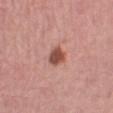Q: Is there a histopathology result?
A: imaged on a skin check; not biopsied
Q: What kind of image is this?
A: 15 mm crop, total-body photography
Q: Who is the patient?
A: female, aged approximately 45
Q: Lesion size?
A: ~2.5 mm (longest diameter)
Q: What is the anatomic site?
A: the left lower leg
Q: Automated lesion metrics?
A: a footprint of about 4.5 mm², an eccentricity of roughly 0.5, and a symmetry-axis asymmetry near 0.25; an average lesion color of about L≈50 a*≈26 b*≈27 (CIELAB), about 15 CIELAB-L* units darker than the surrounding skin, and a lesion-to-skin contrast of about 10 (normalized; higher = more distinct); a classifier nevus-likeness of about 95/100 and lesion-presence confidence of about 100/100
Q: How was the tile lit?
A: white-light illumination